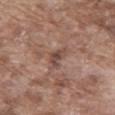Part of a total-body skin-imaging series; this lesion was reviewed on a skin check and was not flagged for biopsy.
On the abdomen.
Captured under white-light illumination.
Approximately 3 mm at its widest.
This image is a 15 mm lesion crop taken from a total-body photograph.
A male patient, aged 73 to 77.